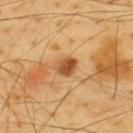biopsy status: catalogued during a skin exam; not biopsied | site: the back | imaging modality: ~15 mm tile from a whole-body skin photo | subject: male, roughly 65 years of age | lesion diameter: ≈3 mm | lighting: cross-polarized | automated metrics: an area of roughly 4.5 mm² and an outline eccentricity of about 0.55 (0 = round, 1 = elongated); a within-lesion color-variation index near 4/10 and peripheral color asymmetry of about 1.5.